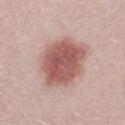This lesion was catalogued during total-body skin photography and was not selected for biopsy. A male subject, aged 28–32. A 15 mm close-up extracted from a 3D total-body photography capture. Approximately 7 mm at its widest. Automated image analysis of the tile measured a mean CIELAB color near L≈55 a*≈24 b*≈24 and a lesion–skin lightness drop of about 15.